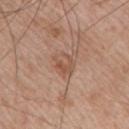biopsy status = no biopsy performed (imaged during a skin exam) | body site = the upper back | lighting = white-light illumination | lesion size = about 3 mm | automated metrics = an average lesion color of about L≈53 a*≈21 b*≈30 (CIELAB), about 8 CIELAB-L* units darker than the surrounding skin, and a normalized lesion–skin contrast near 6; a lesion-detection confidence of about 100/100 | imaging modality = 15 mm crop, total-body photography | subject = male, roughly 65 years of age.